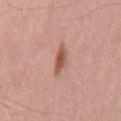Q: Was a biopsy performed?
A: total-body-photography surveillance lesion; no biopsy
Q: What are the patient's age and sex?
A: male, aged 63 to 67
Q: What kind of image is this?
A: 15 mm crop, total-body photography
Q: How large is the lesion?
A: ~3.5 mm (longest diameter)
Q: What is the anatomic site?
A: the mid back
Q: What lighting was used for the tile?
A: white-light
Q: Automated lesion metrics?
A: roughly 12 lightness units darker than nearby skin; a nevus-likeness score of about 100/100 and a lesion-detection confidence of about 100/100